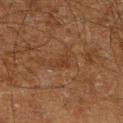Q: Was a biopsy performed?
A: no biopsy performed (imaged during a skin exam)
Q: Automated lesion metrics?
A: an area of roughly 3 mm², an eccentricity of roughly 0.85, and a symmetry-axis asymmetry near 0.4; a border-irregularity rating of about 4/10 and a color-variation rating of about 0.5/10
Q: What are the patient's age and sex?
A: male, in their 60s
Q: How was the tile lit?
A: cross-polarized illumination
Q: Where on the body is the lesion?
A: the right lower leg
Q: What is the lesion's diameter?
A: ~3 mm (longest diameter)
Q: What kind of image is this?
A: ~15 mm crop, total-body skin-cancer survey